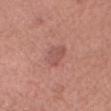Imaged during a routine full-body skin examination; the lesion was not biopsied and no histopathology is available. The lesion is on the head or neck. A female patient in their 40s. A 15 mm close-up extracted from a 3D total-body photography capture.